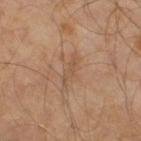<tbp_lesion>
<biopsy_status>not biopsied; imaged during a skin examination</biopsy_status>
<site>leg</site>
<image>
  <source>total-body photography crop</source>
  <field_of_view_mm>15</field_of_view_mm>
</image>
<patient>
  <sex>male</sex>
  <age_approx>60</age_approx>
</patient>
</tbp_lesion>